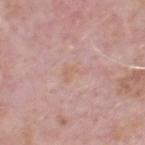Notes:
– follow-up: imaged on a skin check; not biopsied
– size: about 3.5 mm
– location: the head or neck
– patient: male, about 70 years old
– image: total-body-photography crop, ~15 mm field of view
– tile lighting: white-light illumination